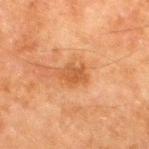Impression: The lesion was photographed on a routine skin check and not biopsied; there is no pathology result. Background: An algorithmic analysis of the crop reported an area of roughly 4.5 mm², an outline eccentricity of about 0.7 (0 = round, 1 = elongated), and a shape-asymmetry score of about 0.3 (0 = symmetric). The software also gave border irregularity of about 3 on a 0–10 scale and peripheral color asymmetry of about 0.5. A lesion tile, about 15 mm wide, cut from a 3D total-body photograph. This is a cross-polarized tile. The lesion is on the right thigh. The lesion's longest dimension is about 2.5 mm. The patient is a male aged approximately 80.